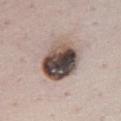Impression:
This lesion was catalogued during total-body skin photography and was not selected for biopsy.
Background:
Measured at roughly 5.5 mm in maximum diameter. A region of skin cropped from a whole-body photographic capture, roughly 15 mm wide. The lesion is located on the chest. A female patient aged approximately 50. An algorithmic analysis of the crop reported a lesion–skin lightness drop of about 22 and a normalized border contrast of about 14.5. The analysis additionally found border irregularity of about 1 on a 0–10 scale, a within-lesion color-variation index near 10/10, and a peripheral color-asymmetry measure near 6. The software also gave a classifier nevus-likeness of about 20/100. This is a white-light tile.